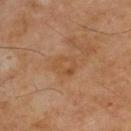  biopsy_status: not biopsied; imaged during a skin examination
  patient:
    sex: male
    age_approx: 55
  site: upper back
  image:
    source: total-body photography crop
    field_of_view_mm: 15
  lesion_size:
    long_diameter_mm_approx: 3.0
  lighting: cross-polarized